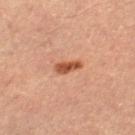This lesion was catalogued during total-body skin photography and was not selected for biopsy.
A female subject aged 58 to 62.
The lesion is located on the leg.
A 15 mm crop from a total-body photograph taken for skin-cancer surveillance.
Captured under cross-polarized illumination.